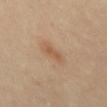biopsy_status: not biopsied; imaged during a skin examination
image:
  source: total-body photography crop
  field_of_view_mm: 15
site: abdomen
lighting: cross-polarized
automated_metrics:
  area_mm2_approx: 3.0
  eccentricity: 0.95
  shape_asymmetry: 0.3
  vs_skin_contrast_norm: 6.0
  color_variation_0_10: 0.0
patient:
  sex: female
  age_approx: 50
lesion_size:
  long_diameter_mm_approx: 3.0